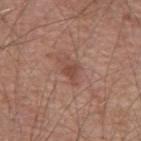Clinical impression: The lesion was photographed on a routine skin check and not biopsied; there is no pathology result.